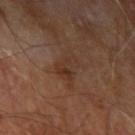location: the left upper arm
patient: male, aged approximately 70
acquisition: ~15 mm crop, total-body skin-cancer survey
lesion diameter: about 4 mm
automated metrics: an area of roughly 7 mm²; an average lesion color of about L≈33 a*≈18 b*≈26 (CIELAB) and a normalized lesion–skin contrast near 5.5